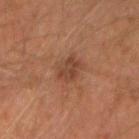Acquisition and patient details: Located on the left forearm. The lesion-visualizer software estimated a mean CIELAB color near L≈34 a*≈19 b*≈26, about 7 CIELAB-L* units darker than the surrounding skin, and a normalized lesion–skin contrast near 6.5. It also reported a nevus-likeness score of about 10/100 and lesion-presence confidence of about 100/100. A male patient, aged 43–47. A 15 mm close-up extracted from a 3D total-body photography capture. Captured under cross-polarized illumination.